On the mid back · a close-up tile cropped from a whole-body skin photograph, about 15 mm across · a female patient, aged around 50:
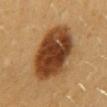{"automated_metrics": {"eccentricity": 0.75, "shape_asymmetry": 0.15, "cielab_L": 43, "cielab_a": 22, "cielab_b": 37, "vs_skin_darker_L": 18.0, "vs_skin_contrast_norm": 13.0, "nevus_likeness_0_100": 100}, "lesion_size": {"long_diameter_mm_approx": 8.0}, "lighting": "cross-polarized", "diagnosis": {"histopathology": "dysplastic (Clark) nevus", "malignancy": "benign", "taxonomic_path": ["Benign", "Benign melanocytic proliferations", "Nevus", "Nevus, Atypical, Dysplastic, or Clark"]}}